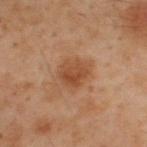No biopsy was performed on this lesion — it was imaged during a full skin examination and was not determined to be concerning. The lesion-visualizer software estimated a shape eccentricity near 0.35. The analysis additionally found a mean CIELAB color near L≈39 a*≈19 b*≈29, about 7 CIELAB-L* units darker than the surrounding skin, and a normalized lesion–skin contrast near 7. The analysis additionally found lesion-presence confidence of about 100/100. A 15 mm close-up tile from a total-body photography series done for melanoma screening. The recorded lesion diameter is about 3.5 mm. Located on the upper back. A male patient roughly 50 years of age.